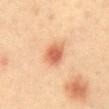{
  "biopsy_status": "not biopsied; imaged during a skin examination",
  "lesion_size": {
    "long_diameter_mm_approx": 3.0
  },
  "automated_metrics": {
    "area_mm2_approx": 7.0,
    "eccentricity": 0.55,
    "cielab_L": 57,
    "cielab_a": 25,
    "cielab_b": 34,
    "vs_skin_darker_L": 12.0,
    "vs_skin_contrast_norm": 8.0
  },
  "lighting": "cross-polarized",
  "site": "abdomen",
  "image": {
    "source": "total-body photography crop",
    "field_of_view_mm": 15
  },
  "patient": {
    "sex": "male",
    "age_approx": 40
  }
}